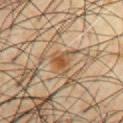notes: imaged on a skin check; not biopsied | imaging modality: ~15 mm crop, total-body skin-cancer survey | size: ~2.5 mm (longest diameter) | illumination: cross-polarized | location: the front of the torso | automated lesion analysis: an area of roughly 4.5 mm², an outline eccentricity of about 0.55 (0 = round, 1 = elongated), and two-axis asymmetry of about 0.35; a mean CIELAB color near L≈51 a*≈19 b*≈36, a lesion–skin lightness drop of about 9, and a normalized border contrast of about 8; border irregularity of about 4 on a 0–10 scale, internal color variation of about 3.5 on a 0–10 scale, and a peripheral color-asymmetry measure near 1 | patient: male, aged around 60.